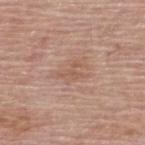follow-up — imaged on a skin check; not biopsied
patient — male, approximately 80 years of age
site — the upper back
lesion diameter — about 3 mm
image source — total-body-photography crop, ~15 mm field of view
tile lighting — white-light illumination
image-analysis metrics — an average lesion color of about L≈56 a*≈20 b*≈29 (CIELAB), about 6 CIELAB-L* units darker than the surrounding skin, and a normalized lesion–skin contrast near 5; a border-irregularity index near 7.5/10, internal color variation of about 0 on a 0–10 scale, and a peripheral color-asymmetry measure near 0; an automated nevus-likeness rating near 0 out of 100 and a detector confidence of about 100 out of 100 that the crop contains a lesion